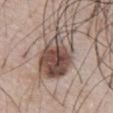Impression:
The lesion was tiled from a total-body skin photograph and was not biopsied.
Clinical summary:
The total-body-photography lesion software estimated a lesion area of about 23 mm², an eccentricity of roughly 0.85, and a symmetry-axis asymmetry near 0.2. The analysis additionally found a border-irregularity index near 3/10 and radial color variation of about 4. And it measured a nevus-likeness score of about 100/100 and lesion-presence confidence of about 95/100. A close-up tile cropped from a whole-body skin photograph, about 15 mm across. On the front of the torso. A male patient, about 70 years old. Measured at roughly 7.5 mm in maximum diameter. Imaged with white-light lighting.